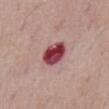Clinical impression:
Captured during whole-body skin photography for melanoma surveillance; the lesion was not biopsied.
Image and clinical context:
A 15 mm crop from a total-body photograph taken for skin-cancer surveillance. Located on the abdomen. About 4 mm across. A male subject, about 70 years old.